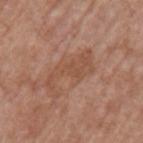Recorded during total-body skin imaging; not selected for excision or biopsy.
The recorded lesion diameter is about 6 mm.
A roughly 15 mm field-of-view crop from a total-body skin photograph.
From the left upper arm.
A male subject, approximately 75 years of age.
Imaged with white-light lighting.